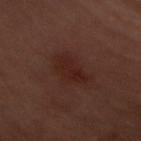Impression:
This lesion was catalogued during total-body skin photography and was not selected for biopsy.
Image and clinical context:
The patient is a female aged approximately 60. On the back. A region of skin cropped from a whole-body photographic capture, roughly 15 mm wide.